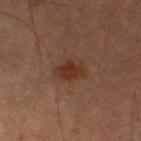<tbp_lesion>
  <biopsy_status>not biopsied; imaged during a skin examination</biopsy_status>
  <lesion_size>
    <long_diameter_mm_approx>3.5</long_diameter_mm_approx>
  </lesion_size>
  <patient>
    <sex>male</sex>
    <age_approx>75</age_approx>
  </patient>
  <image>
    <source>total-body photography crop</source>
    <field_of_view_mm>15</field_of_view_mm>
  </image>
  <lighting>cross-polarized</lighting>
  <site>left thigh</site>
</tbp_lesion>